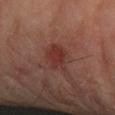Case summary:
– follow-up: total-body-photography surveillance lesion; no biopsy
– patient: male, roughly 70 years of age
– acquisition: ~15 mm crop, total-body skin-cancer survey
– anatomic site: the left forearm